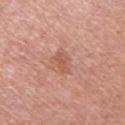* size — ≈3 mm
* body site — the right upper arm
* tile lighting — white-light
* image — total-body-photography crop, ~15 mm field of view
* patient — female, approximately 40 years of age